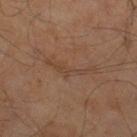Clinical impression: Part of a total-body skin-imaging series; this lesion was reviewed on a skin check and was not flagged for biopsy. Image and clinical context: The lesion is on the right thigh. Cropped from a total-body skin-imaging series; the visible field is about 15 mm. Imaged with cross-polarized lighting. The patient is a male aged 58 to 62. Approximately 6 mm at its widest.